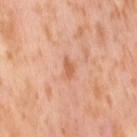Case summary:
* biopsy status: no biopsy performed (imaged during a skin exam)
* image-analysis metrics: a footprint of about 2.5 mm², an outline eccentricity of about 0.9 (0 = round, 1 = elongated), and a shape-asymmetry score of about 0.4 (0 = symmetric); a lesion–skin lightness drop of about 9 and a lesion-to-skin contrast of about 6.5 (normalized; higher = more distinct); border irregularity of about 4 on a 0–10 scale, internal color variation of about 0 on a 0–10 scale, and a peripheral color-asymmetry measure near 0
* subject: female, in their mid-50s
* image: ~15 mm tile from a whole-body skin photo
* illumination: cross-polarized
* anatomic site: the right thigh